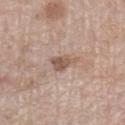Q: Was a biopsy performed?
A: no biopsy performed (imaged during a skin exam)
Q: Patient demographics?
A: male, aged around 70
Q: What kind of image is this?
A: 15 mm crop, total-body photography
Q: Where on the body is the lesion?
A: the left lower leg